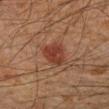Assessment:
Imaged during a routine full-body skin examination; the lesion was not biopsied and no histopathology is available.
Context:
This is a cross-polarized tile. From the left forearm. A close-up tile cropped from a whole-body skin photograph, about 15 mm across. Longest diameter approximately 3 mm. A male patient aged around 50.